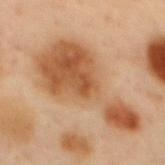Clinical impression: Imaged during a routine full-body skin examination; the lesion was not biopsied and no histopathology is available.